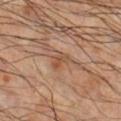follow-up: total-body-photography surveillance lesion; no biopsy
location: the right lower leg
image source: ~15 mm tile from a whole-body skin photo
lighting: cross-polarized illumination
patient: male, roughly 60 years of age
diameter: about 3 mm